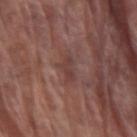anatomic site = the right upper arm | image = ~15 mm tile from a whole-body skin photo | lesion size = ~3 mm (longest diameter) | subject = male, approximately 80 years of age | automated lesion analysis = a lesion color around L≈39 a*≈20 b*≈21 in CIELAB, roughly 6 lightness units darker than nearby skin, and a normalized border contrast of about 5.5; a lesion-detection confidence of about 55/100.